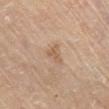Findings:
• notes — catalogued during a skin exam; not biopsied
• TBP lesion metrics — an area of roughly 3.5 mm², an outline eccentricity of about 0.65 (0 = round, 1 = elongated), and a symmetry-axis asymmetry near 0.55; roughly 8 lightness units darker than nearby skin and a lesion-to-skin contrast of about 5.5 (normalized; higher = more distinct); a classifier nevus-likeness of about 0/100 and a detector confidence of about 100 out of 100 that the crop contains a lesion
• diameter — ~2.5 mm (longest diameter)
• body site — the chest
• lighting — white-light
• image — total-body-photography crop, ~15 mm field of view
• subject — male, aged approximately 80